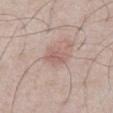{"biopsy_status": "not biopsied; imaged during a skin examination", "patient": {"sex": "male", "age_approx": 60}, "site": "front of the torso", "lighting": "white-light", "automated_metrics": {"area_mm2_approx": 5.0, "eccentricity": 0.8, "shape_asymmetry": 0.3, "cielab_L": 59, "cielab_a": 19, "cielab_b": 23, "vs_skin_darker_L": 8.0, "vs_skin_contrast_norm": 5.5, "border_irregularity_0_10": 3.0, "peripheral_color_asymmetry": 1.0, "lesion_detection_confidence_0_100": 100}, "lesion_size": {"long_diameter_mm_approx": 3.0}, "image": {"source": "total-body photography crop", "field_of_view_mm": 15}}